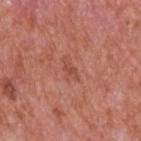This lesion was catalogued during total-body skin photography and was not selected for biopsy. On the upper back. Automated tile analysis of the lesion measured a lesion area of about 2.5 mm², a shape eccentricity near 0.85, and two-axis asymmetry of about 0.45. The software also gave a lesion color around L≈49 a*≈28 b*≈29 in CIELAB and roughly 7 lightness units darker than nearby skin. The software also gave a border-irregularity index near 4.5/10, a within-lesion color-variation index near 0/10, and a peripheral color-asymmetry measure near 0. It also reported a classifier nevus-likeness of about 0/100 and lesion-presence confidence of about 100/100. A 15 mm crop from a total-body photograph taken for skin-cancer surveillance. Captured under white-light illumination. The patient is a male aged approximately 65. Approximately 2.5 mm at its widest.